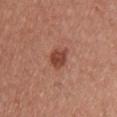A male subject, in their 40s.
Captured under white-light illumination.
The lesion is on the upper back.
A 15 mm close-up tile from a total-body photography series done for melanoma screening.
Measured at roughly 3 mm in maximum diameter.